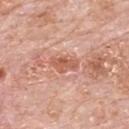| key | value |
|---|---|
| workup | catalogued during a skin exam; not biopsied |
| acquisition | 15 mm crop, total-body photography |
| automated metrics | an area of roughly 5 mm², a shape eccentricity near 0.9, and a shape-asymmetry score of about 0.25 (0 = symmetric); a lesion color around L≈58 a*≈27 b*≈31 in CIELAB and a normalized border contrast of about 7.5; a border-irregularity index near 3/10, a color-variation rating of about 3/10, and a peripheral color-asymmetry measure near 1 |
| patient | male, roughly 80 years of age |
| illumination | white-light illumination |
| lesion diameter | ~3.5 mm (longest diameter) |
| body site | the mid back |